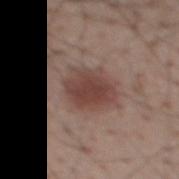This lesion was catalogued during total-body skin photography and was not selected for biopsy.
The lesion is on the mid back.
About 4.5 mm across.
A male subject aged 58–62.
A region of skin cropped from a whole-body photographic capture, roughly 15 mm wide.